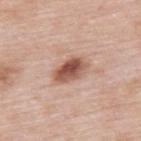Imaged during a routine full-body skin examination; the lesion was not biopsied and no histopathology is available. Automated tile analysis of the lesion measured an average lesion color of about L≈54 a*≈23 b*≈28 (CIELAB), a lesion–skin lightness drop of about 15, and a normalized border contrast of about 10. A male patient aged around 55. The lesion is on the upper back. A close-up tile cropped from a whole-body skin photograph, about 15 mm across. The recorded lesion diameter is about 4 mm. Imaged with white-light lighting.